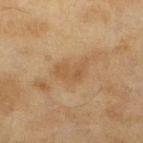notes = catalogued during a skin exam; not biopsied
image source = ~15 mm crop, total-body skin-cancer survey
automated metrics = a mean CIELAB color near L≈50 a*≈17 b*≈34; a border-irregularity rating of about 5/10 and radial color variation of about 0.5; an automated nevus-likeness rating near 0 out of 100 and a lesion-detection confidence of about 100/100
illumination = cross-polarized illumination
site = the left lower leg
subject = female, roughly 60 years of age
lesion size = ~4 mm (longest diameter)